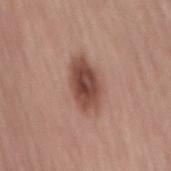notes — catalogued during a skin exam; not biopsied | lighting — white-light illumination | lesion diameter — ≈5 mm | patient — female, aged 63–67 | image — ~15 mm tile from a whole-body skin photo | location — the mid back.